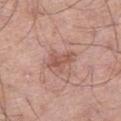| feature | finding |
|---|---|
| notes | total-body-photography surveillance lesion; no biopsy |
| size | about 3.5 mm |
| automated lesion analysis | a lesion–skin lightness drop of about 9 |
| location | the leg |
| tile lighting | white-light illumination |
| imaging modality | ~15 mm tile from a whole-body skin photo |
| subject | male, in their 70s |